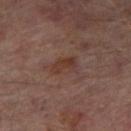<lesion>
<biopsy_status>not biopsied; imaged during a skin examination</biopsy_status>
<image>
  <source>total-body photography crop</source>
  <field_of_view_mm>15</field_of_view_mm>
</image>
<lighting>cross-polarized</lighting>
<patient>
  <sex>male</sex>
  <age_approx>65</age_approx>
</patient>
<lesion_size>
  <long_diameter_mm_approx>3.0</long_diameter_mm_approx>
</lesion_size>
<site>right thigh</site>
</lesion>